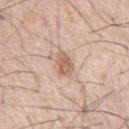Q: Was a biopsy performed?
A: catalogued during a skin exam; not biopsied
Q: Illumination type?
A: white-light
Q: What is the imaging modality?
A: ~15 mm tile from a whole-body skin photo
Q: What are the patient's age and sex?
A: male, aged 53–57